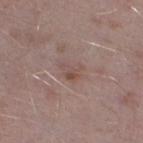Assessment:
Part of a total-body skin-imaging series; this lesion was reviewed on a skin check and was not flagged for biopsy.